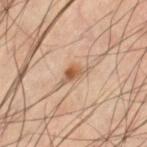Assessment:
The lesion was photographed on a routine skin check and not biopsied; there is no pathology result.
Context:
Located on the left thigh. The tile uses cross-polarized illumination. The total-body-photography lesion software estimated an average lesion color of about L≈55 a*≈19 b*≈33 (CIELAB) and a lesion–skin lightness drop of about 11. And it measured border irregularity of about 4 on a 0–10 scale, a within-lesion color-variation index near 5/10, and a peripheral color-asymmetry measure near 2. A 15 mm close-up tile from a total-body photography series done for melanoma screening. A male subject, aged 58–62. The recorded lesion diameter is about 3.5 mm.